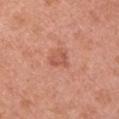Recorded during total-body skin imaging; not selected for excision or biopsy. A 15 mm crop from a total-body photograph taken for skin-cancer surveillance. Located on the left upper arm. A female patient approximately 40 years of age.